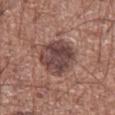Imaged during a routine full-body skin examination; the lesion was not biopsied and no histopathology is available. The lesion is located on the chest. The subject is a male in their mid- to late 70s. A region of skin cropped from a whole-body photographic capture, roughly 15 mm wide.